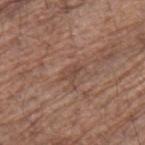Clinical impression: The lesion was photographed on a routine skin check and not biopsied; there is no pathology result. Context: The lesion is on the upper back. Cropped from a total-body skin-imaging series; the visible field is about 15 mm. The total-body-photography lesion software estimated a mean CIELAB color near L≈46 a*≈19 b*≈25, a lesion–skin lightness drop of about 7, and a lesion-to-skin contrast of about 5 (normalized; higher = more distinct). It also reported border irregularity of about 3 on a 0–10 scale, internal color variation of about 1 on a 0–10 scale, and peripheral color asymmetry of about 0.5. This is a white-light tile. Approximately 2.5 mm at its widest. The patient is a male aged 68 to 72.